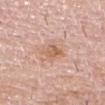follow-up=no biopsy performed (imaged during a skin exam); automated lesion analysis=a mean CIELAB color near L≈62 a*≈21 b*≈32 and roughly 8 lightness units darker than nearby skin; patient=male, aged approximately 80; site=the chest; acquisition=15 mm crop, total-body photography; lighting=white-light illumination.